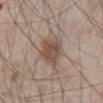Cropped from a total-body skin-imaging series; the visible field is about 15 mm.
Located on the abdomen.
The subject is a male in their mid-60s.